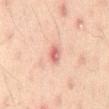biopsy status: catalogued during a skin exam; not biopsied
lesion diameter: ≈2.5 mm
illumination: cross-polarized illumination
body site: the back
subject: male, in their 60s
image: ~15 mm tile from a whole-body skin photo